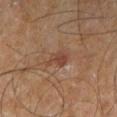workup: no biopsy performed (imaged during a skin exam) | image: ~15 mm tile from a whole-body skin photo | patient: male, roughly 60 years of age | body site: the right leg | tile lighting: cross-polarized | image-analysis metrics: a lesion color around L≈41 a*≈20 b*≈27 in CIELAB, about 7 CIELAB-L* units darker than the surrounding skin, and a lesion-to-skin contrast of about 6.5 (normalized; higher = more distinct); a lesion-detection confidence of about 100/100 | lesion diameter: about 3 mm.